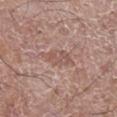* notes — total-body-photography surveillance lesion; no biopsy
* tile lighting — white-light illumination
* lesion size — ≈4 mm
* automated lesion analysis — a shape eccentricity near 0.85 and a shape-asymmetry score of about 0.25 (0 = symmetric); about 7 CIELAB-L* units darker than the surrounding skin and a lesion-to-skin contrast of about 5 (normalized; higher = more distinct); a classifier nevus-likeness of about 0/100
* patient — male, aged 58 to 62
* imaging modality — 15 mm crop, total-body photography
* site — the right lower leg